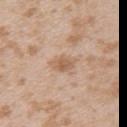biopsy status = imaged on a skin check; not biopsied | body site = the left upper arm | size = ≈3.5 mm | automated metrics = a lesion color around L≈60 a*≈18 b*≈31 in CIELAB, roughly 9 lightness units darker than nearby skin, and a normalized border contrast of about 6 | patient = female, about 25 years old | imaging modality = 15 mm crop, total-body photography.